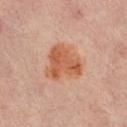biopsy_status: not biopsied; imaged during a skin examination
lesion_size:
  long_diameter_mm_approx: 5.0
site: left thigh
patient:
  sex: female
  age_approx: 50
automated_metrics:
  area_mm2_approx: 17.0
  eccentricity: 0.55
  shape_asymmetry: 0.3
  border_irregularity_0_10: 3.0
  color_variation_0_10: 5.0
  peripheral_color_asymmetry: 1.5
  lesion_detection_confidence_0_100: 100
image:
  source: total-body photography crop
  field_of_view_mm: 15